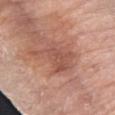Imaged during a routine full-body skin examination; the lesion was not biopsied and no histopathology is available.
A 15 mm close-up extracted from a 3D total-body photography capture.
This is a white-light tile.
A female subject, in their mid- to late 60s.
On the left forearm.
An algorithmic analysis of the crop reported an average lesion color of about L≈53 a*≈24 b*≈28 (CIELAB), about 8 CIELAB-L* units darker than the surrounding skin, and a lesion-to-skin contrast of about 6 (normalized; higher = more distinct). And it measured a nevus-likeness score of about 0/100 and a lesion-detection confidence of about 95/100.